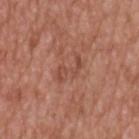Impression: Part of a total-body skin-imaging series; this lesion was reviewed on a skin check and was not flagged for biopsy.